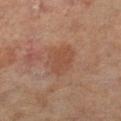biopsy status: catalogued during a skin exam; not biopsied | imaging modality: ~15 mm tile from a whole-body skin photo | lesion diameter: about 4.5 mm | tile lighting: cross-polarized illumination | automated metrics: a footprint of about 10 mm² and an eccentricity of roughly 0.75; a detector confidence of about 100 out of 100 that the crop contains a lesion | anatomic site: the leg | patient: female, in their mid-60s.